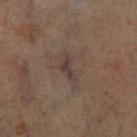<lesion>
  <site>right lower leg</site>
  <image>
    <source>total-body photography crop</source>
    <field_of_view_mm>15</field_of_view_mm>
  </image>
</lesion>